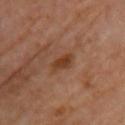follow-up = catalogued during a skin exam; not biopsied
image source = total-body-photography crop, ~15 mm field of view
subject = female, aged around 60
TBP lesion metrics = a lesion color around L≈41 a*≈23 b*≈34 in CIELAB and a normalized border contrast of about 8
size = ≈3 mm
location = the front of the torso